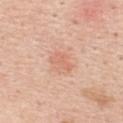follow-up: total-body-photography surveillance lesion; no biopsy
acquisition: total-body-photography crop, ~15 mm field of view
subject: female, roughly 45 years of age
anatomic site: the upper back
image-analysis metrics: an area of roughly 3.5 mm², a shape eccentricity near 0.8, and two-axis asymmetry of about 0.55; a mean CIELAB color near L≈66 a*≈25 b*≈32 and a lesion-to-skin contrast of about 4.5 (normalized; higher = more distinct)